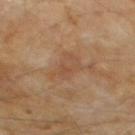Findings:
– workup: imaged on a skin check; not biopsied
– image source: 15 mm crop, total-body photography
– tile lighting: cross-polarized illumination
– subject: male, aged approximately 60
– body site: the chest
– lesion diameter: about 4.5 mm